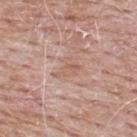No biopsy was performed on this lesion — it was imaged during a full skin examination and was not determined to be concerning. An algorithmic analysis of the crop reported border irregularity of about 6 on a 0–10 scale, a within-lesion color-variation index near 3.5/10, and peripheral color asymmetry of about 1. It also reported a classifier nevus-likeness of about 0/100. The lesion is located on the mid back. The lesion's longest dimension is about 3.5 mm. The tile uses white-light illumination. A lesion tile, about 15 mm wide, cut from a 3D total-body photograph. A male subject roughly 65 years of age.